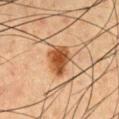biopsy status=total-body-photography surveillance lesion; no biopsy | patient=male, approximately 50 years of age | lesion size=≈4 mm | image-analysis metrics=an average lesion color of about L≈40 a*≈21 b*≈32 (CIELAB) and a lesion–skin lightness drop of about 13; border irregularity of about 3 on a 0–10 scale and a color-variation rating of about 3/10 | location=the chest | acquisition=~15 mm tile from a whole-body skin photo | illumination=cross-polarized illumination.